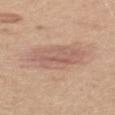Case summary:
* notes — total-body-photography surveillance lesion; no biopsy
* subject — female, aged around 55
* TBP lesion metrics — a mean CIELAB color near L≈59 a*≈20 b*≈26; a border-irregularity rating of about 2.5/10, internal color variation of about 4 on a 0–10 scale, and radial color variation of about 1.5
* location — the upper back
* image — ~15 mm crop, total-body skin-cancer survey
* tile lighting — white-light
* size — ≈6 mm